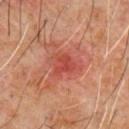A male subject in their 60s. The total-body-photography lesion software estimated an area of roughly 5 mm² and an eccentricity of roughly 0.6. A lesion tile, about 15 mm wide, cut from a 3D total-body photograph. Approximately 3 mm at its widest. Located on the chest.